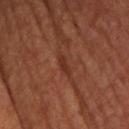Impression: The lesion was tiled from a total-body skin photograph and was not biopsied. Acquisition and patient details: The lesion is located on the chest. A 15 mm close-up tile from a total-body photography series done for melanoma screening. Approximately 2.5 mm at its widest. The patient is a male about 65 years old.